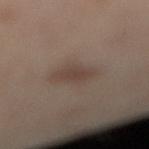Q: Was a biopsy performed?
A: imaged on a skin check; not biopsied
Q: What kind of image is this?
A: ~15 mm tile from a whole-body skin photo
Q: Illumination type?
A: cross-polarized
Q: What is the anatomic site?
A: the left leg
Q: What are the patient's age and sex?
A: female, in their 40s
Q: What did automated image analysis measure?
A: an area of roughly 7.5 mm², an eccentricity of roughly 0.7, and a symmetry-axis asymmetry near 0.2; about 7 CIELAB-L* units darker than the surrounding skin and a lesion-to-skin contrast of about 6 (normalized; higher = more distinct)